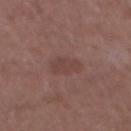Notes:
• follow-up: imaged on a skin check; not biopsied
• patient: male, in their mid- to late 50s
• image: total-body-photography crop, ~15 mm field of view
• site: the abdomen
• diameter: ~3 mm (longest diameter)
• automated metrics: a lesion area of about 6 mm² and an outline eccentricity of about 0.45 (0 = round, 1 = elongated); an average lesion color of about L≈42 a*≈20 b*≈21 (CIELAB) and a normalized lesion–skin contrast near 5; a border-irregularity rating of about 3/10 and peripheral color asymmetry of about 0.5